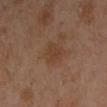Captured during whole-body skin photography for melanoma surveillance; the lesion was not biopsied. The patient is a female approximately 40 years of age. From the arm. Automated tile analysis of the lesion measured a lesion–skin lightness drop of about 5 and a normalized border contrast of about 5.5. A roughly 15 mm field-of-view crop from a total-body skin photograph. Captured under cross-polarized illumination.